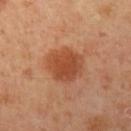Clinical impression:
Captured during whole-body skin photography for melanoma surveillance; the lesion was not biopsied.
Context:
From the left upper arm. A 15 mm close-up extracted from a 3D total-body photography capture. Captured under cross-polarized illumination. A female patient about 40 years old.